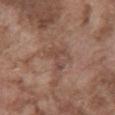Recorded during total-body skin imaging; not selected for excision or biopsy. A region of skin cropped from a whole-body photographic capture, roughly 15 mm wide. The tile uses white-light illumination. A male patient in their mid-70s. The recorded lesion diameter is about 3 mm. The lesion is located on the abdomen.